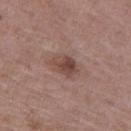Q: Was this lesion biopsied?
A: catalogued during a skin exam; not biopsied
Q: How was the tile lit?
A: white-light
Q: Lesion size?
A: about 3.5 mm
Q: Who is the patient?
A: male, roughly 70 years of age
Q: Where on the body is the lesion?
A: the left thigh
Q: How was this image acquired?
A: 15 mm crop, total-body photography